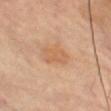workup = no biopsy performed (imaged during a skin exam) | imaging modality = ~15 mm tile from a whole-body skin photo | tile lighting = cross-polarized | lesion diameter = ~4 mm (longest diameter) | subject = female, aged 78–82 | site = the right leg.